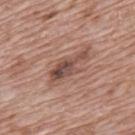{"biopsy_status": "not biopsied; imaged during a skin examination", "lighting": "white-light", "lesion_size": {"long_diameter_mm_approx": 5.0}, "automated_metrics": {"area_mm2_approx": 7.0, "cielab_L": 48, "cielab_a": 20, "cielab_b": 24, "vs_skin_darker_L": 12.0, "color_variation_0_10": 4.5}, "site": "back", "patient": {"sex": "male", "age_approx": 70}, "image": {"source": "total-body photography crop", "field_of_view_mm": 15}}